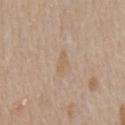Captured during whole-body skin photography for melanoma surveillance; the lesion was not biopsied.
The lesion is on the chest.
A roughly 15 mm field-of-view crop from a total-body skin photograph.
The tile uses white-light illumination.
A male patient, in their mid-60s.
The total-body-photography lesion software estimated a lesion area of about 2.5 mm², an outline eccentricity of about 0.9 (0 = round, 1 = elongated), and two-axis asymmetry of about 0.35. The analysis additionally found a lesion color around L≈60 a*≈15 b*≈31 in CIELAB, about 6 CIELAB-L* units darker than the surrounding skin, and a normalized border contrast of about 5. It also reported an automated nevus-likeness rating near 0 out of 100 and a detector confidence of about 100 out of 100 that the crop contains a lesion.
Measured at roughly 2.5 mm in maximum diameter.